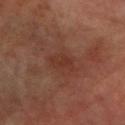<lesion>
<biopsy_status>not biopsied; imaged during a skin examination</biopsy_status>
<image>
  <source>total-body photography crop</source>
  <field_of_view_mm>15</field_of_view_mm>
</image>
<lighting>cross-polarized</lighting>
<site>arm</site>
<patient>
  <sex>male</sex>
  <age_approx>70</age_approx>
</patient>
<automated_metrics>
  <border_irregularity_0_10>3.0</border_irregularity_0_10>
  <color_variation_0_10>2.0</color_variation_0_10>
  <peripheral_color_asymmetry>0.5</peripheral_color_asymmetry>
</automated_metrics>
<lesion_size>
  <long_diameter_mm_approx>3.0</long_diameter_mm_approx>
</lesion_size>
</lesion>